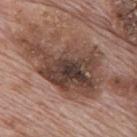Q: Is there a histopathology result?
A: no biopsy performed (imaged during a skin exam)
Q: How large is the lesion?
A: ≈9.5 mm
Q: What are the patient's age and sex?
A: male, roughly 70 years of age
Q: What is the imaging modality?
A: total-body-photography crop, ~15 mm field of view
Q: What is the anatomic site?
A: the mid back